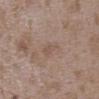workup: catalogued during a skin exam; not biopsied
automated metrics: a mean CIELAB color near L≈52 a*≈16 b*≈25, a lesion–skin lightness drop of about 6, and a lesion-to-skin contrast of about 4.5 (normalized; higher = more distinct)
tile lighting: white-light illumination
image source: ~15 mm tile from a whole-body skin photo
anatomic site: the upper back
patient: female, roughly 35 years of age
size: ~2.5 mm (longest diameter)